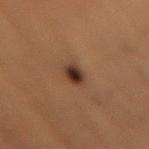The lesion was photographed on a routine skin check and not biopsied; there is no pathology result.
This is a cross-polarized tile.
Approximately 2.5 mm at its widest.
A male patient, roughly 50 years of age.
Located on the lower back.
A region of skin cropped from a whole-body photographic capture, roughly 15 mm wide.
An algorithmic analysis of the crop reported a lesion area of about 4 mm², an eccentricity of roughly 0.8, and a shape-asymmetry score of about 0.15 (0 = symmetric). It also reported a color-variation rating of about 4/10.